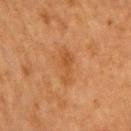imaging modality: total-body-photography crop, ~15 mm field of view
automated lesion analysis: an area of roughly 7 mm² and a symmetry-axis asymmetry near 0.35; an average lesion color of about L≈43 a*≈21 b*≈35 (CIELAB), about 6 CIELAB-L* units darker than the surrounding skin, and a normalized lesion–skin contrast near 5.5; a nevus-likeness score of about 0/100 and a detector confidence of about 100 out of 100 that the crop contains a lesion
tile lighting: cross-polarized illumination
body site: the right upper arm
lesion size: about 4.5 mm
patient: female, aged around 55